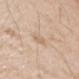notes: total-body-photography surveillance lesion; no biopsy | tile lighting: white-light illumination | body site: the left upper arm | lesion diameter: ≈3 mm | subject: male, aged 63 to 67 | image: 15 mm crop, total-body photography.